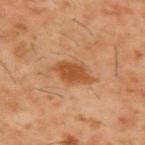  image:
    source: total-body photography crop
    field_of_view_mm: 15
  site: upper back
  patient:
    sex: male
    age_approx: 60